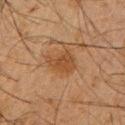Findings:
* workup — no biopsy performed (imaged during a skin exam)
* anatomic site — the left upper arm
* illumination — cross-polarized
* acquisition — ~15 mm crop, total-body skin-cancer survey
* subject — male, aged approximately 35